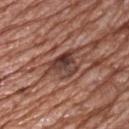{
  "biopsy_status": "not biopsied; imaged during a skin examination",
  "automated_metrics": {
    "cielab_L": 40,
    "cielab_a": 21,
    "cielab_b": 24,
    "vs_skin_darker_L": 11.0,
    "vs_skin_contrast_norm": 9.0,
    "nevus_likeness_0_100": 0,
    "lesion_detection_confidence_0_100": 80
  },
  "image": {
    "source": "total-body photography crop",
    "field_of_view_mm": 15
  },
  "lesion_size": {
    "long_diameter_mm_approx": 5.0
  },
  "patient": {
    "sex": "male",
    "age_approx": 70
  },
  "site": "front of the torso"
}